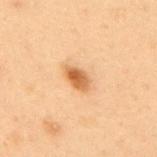| field | value |
|---|---|
| biopsy status | imaged on a skin check; not biopsied |
| illumination | cross-polarized |
| TBP lesion metrics | a lesion area of about 5 mm² and an eccentricity of roughly 0.8; an average lesion color of about L≈51 a*≈20 b*≈36 (CIELAB), a lesion–skin lightness drop of about 12, and a lesion-to-skin contrast of about 9 (normalized; higher = more distinct); a border-irregularity index near 2.5/10 and internal color variation of about 5 on a 0–10 scale |
| lesion diameter | ≈3.5 mm |
| patient | male, aged around 55 |
| anatomic site | the upper back |
| image source | ~15 mm tile from a whole-body skin photo |